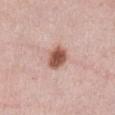Assessment: Captured during whole-body skin photography for melanoma surveillance; the lesion was not biopsied. Context: Measured at roughly 3.5 mm in maximum diameter. This is a white-light tile. The lesion is on the abdomen. Cropped from a total-body skin-imaging series; the visible field is about 15 mm. A female patient, aged 38 to 42.